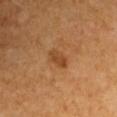{
  "biopsy_status": "not biopsied; imaged during a skin examination",
  "image": {
    "source": "total-body photography crop",
    "field_of_view_mm": 15
  },
  "automated_metrics": {
    "area_mm2_approx": 4.0,
    "eccentricity": 0.85,
    "shape_asymmetry": 0.3,
    "cielab_L": 42,
    "cielab_a": 22,
    "cielab_b": 37,
    "vs_skin_darker_L": 8.0,
    "vs_skin_contrast_norm": 7.0,
    "color_variation_0_10": 2.5,
    "peripheral_color_asymmetry": 1.0,
    "nevus_likeness_0_100": 30
  },
  "lesion_size": {
    "long_diameter_mm_approx": 3.0
  },
  "patient": {
    "sex": "male",
    "age_approx": 60
  },
  "lighting": "cross-polarized",
  "site": "left upper arm"
}